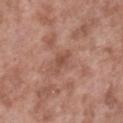{
  "site": "right lower leg",
  "image": {
    "source": "total-body photography crop",
    "field_of_view_mm": 15
  },
  "patient": {
    "sex": "male",
    "age_approx": 55
  }
}